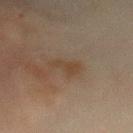Clinical impression: Captured during whole-body skin photography for melanoma surveillance; the lesion was not biopsied. Acquisition and patient details: This is a cross-polarized tile. About 3 mm across. Located on the chest. The patient is a female aged 48–52. A region of skin cropped from a whole-body photographic capture, roughly 15 mm wide. An algorithmic analysis of the crop reported an area of roughly 4 mm², an outline eccentricity of about 0.8 (0 = round, 1 = elongated), and a symmetry-axis asymmetry near 0.4. The analysis additionally found a lesion color around L≈33 a*≈13 b*≈24 in CIELAB, a lesion–skin lightness drop of about 5, and a lesion-to-skin contrast of about 5.5 (normalized; higher = more distinct). The software also gave a within-lesion color-variation index near 1/10 and peripheral color asymmetry of about 0.5.